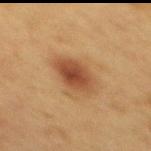The lesion was photographed on a routine skin check and not biopsied; there is no pathology result.
This is a cross-polarized tile.
The patient is a female aged 48 to 52.
A region of skin cropped from a whole-body photographic capture, roughly 15 mm wide.
Located on the mid back.